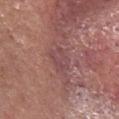Part of a total-body skin-imaging series; this lesion was reviewed on a skin check and was not flagged for biopsy.
Approximately 2.5 mm at its widest.
A 15 mm close-up extracted from a 3D total-body photography capture.
Located on the head or neck.
A male subject, aged 53–57.
The tile uses white-light illumination.